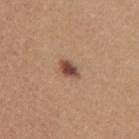follow-up: imaged on a skin check; not biopsied
diameter: ~3 mm (longest diameter)
tile lighting: white-light
imaging modality: 15 mm crop, total-body photography
subject: female, approximately 40 years of age
body site: the mid back